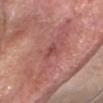Impression: Imaged during a routine full-body skin examination; the lesion was not biopsied and no histopathology is available. Context: A region of skin cropped from a whole-body photographic capture, roughly 15 mm wide. The subject is a female in their mid-60s. The lesion is on the head or neck.